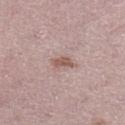Clinical impression: Captured during whole-body skin photography for melanoma surveillance; the lesion was not biopsied. Acquisition and patient details: On the leg. A female subject, aged around 45. The tile uses white-light illumination. The total-body-photography lesion software estimated an area of roughly 3.5 mm², an outline eccentricity of about 0.75 (0 = round, 1 = elongated), and a symmetry-axis asymmetry near 0.3. It also reported a lesion color around L≈56 a*≈19 b*≈23 in CIELAB, about 10 CIELAB-L* units darker than the surrounding skin, and a normalized border contrast of about 7.5. And it measured a classifier nevus-likeness of about 40/100 and a lesion-detection confidence of about 100/100. A close-up tile cropped from a whole-body skin photograph, about 15 mm across.